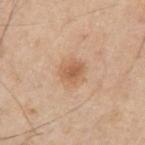site = the right upper arm
imaging modality = ~15 mm tile from a whole-body skin photo
patient = male, aged around 55